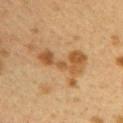The lesion is located on the upper back. A female subject aged 38 to 42. Longest diameter approximately 8.5 mm. The total-body-photography lesion software estimated an area of roughly 17 mm², an outline eccentricity of about 0.9 (0 = round, 1 = elongated), and a symmetry-axis asymmetry near 0.55. And it measured a classifier nevus-likeness of about 5/100 and a detector confidence of about 100 out of 100 that the crop contains a lesion. The tile uses cross-polarized illumination. A 15 mm close-up tile from a total-body photography series done for melanoma screening.